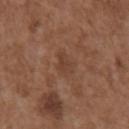  biopsy_status: not biopsied; imaged during a skin examination
  automated_metrics:
    cielab_L: 41
    cielab_a: 20
    cielab_b: 28
    vs_skin_darker_L: 6.0
    vs_skin_contrast_norm: 5.0
    nevus_likeness_0_100: 0
    lesion_detection_confidence_0_100: 100
  site: chest
  patient:
    sex: male
    age_approx: 75
  lighting: white-light
  lesion_size:
    long_diameter_mm_approx: 2.5
  image:
    source: total-body photography crop
    field_of_view_mm: 15